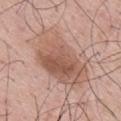workup = total-body-photography surveillance lesion; no biopsy.